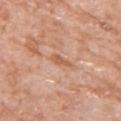The lesion was tiled from a total-body skin photograph and was not biopsied. Captured under white-light illumination. The subject is a female aged approximately 75. An algorithmic analysis of the crop reported a border-irregularity rating of about 4.5/10, a within-lesion color-variation index near 0/10, and peripheral color asymmetry of about 0. A 15 mm close-up extracted from a 3D total-body photography capture. From the upper back.